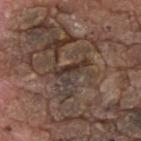Notes:
- biopsy status — imaged on a skin check; not biopsied
- image source — ~15 mm tile from a whole-body skin photo
- subject — male, approximately 75 years of age
- size — ≈4.5 mm
- tile lighting — white-light
- automated lesion analysis — a border-irregularity rating of about 7.5/10 and peripheral color asymmetry of about 1; a classifier nevus-likeness of about 0/100 and a lesion-detection confidence of about 0/100
- anatomic site — the upper back